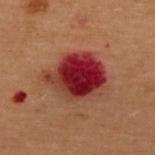Findings:
• notes: imaged on a skin check; not biopsied
• patient: female, in their 50s
• image-analysis metrics: a lesion area of about 23 mm², an outline eccentricity of about 0.6 (0 = round, 1 = elongated), and a symmetry-axis asymmetry near 0.2; a border-irregularity index near 2/10 and a color-variation rating of about 9.5/10
• tile lighting: cross-polarized
• size: about 6 mm
• site: the back
• imaging modality: 15 mm crop, total-body photography